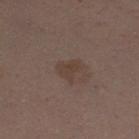Case summary:
– follow-up · no biopsy performed (imaged during a skin exam)
– patient · female, aged 28–32
– image source · ~15 mm crop, total-body skin-cancer survey
– site · the leg